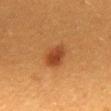biopsy_status: not biopsied; imaged during a skin examination
site: mid back
lighting: cross-polarized
automated_metrics:
  area_mm2_approx: 7.0
  shape_asymmetry: 0.2
  vs_skin_contrast_norm: 8.0
  nevus_likeness_0_100: 100
  lesion_detection_confidence_0_100: 100
image:
  source: total-body photography crop
  field_of_view_mm: 15
patient:
  sex: female
  age_approx: 40
lesion_size:
  long_diameter_mm_approx: 3.0